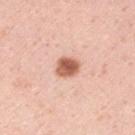  site: left upper arm
  patient:
    sex: male
    age_approx: 35
  lighting: white-light
  image:
    source: total-body photography crop
    field_of_view_mm: 15
  automated_metrics:
    cielab_L: 60
    cielab_a: 25
    cielab_b: 32
    vs_skin_darker_L: 17.0
    vs_skin_contrast_norm: 10.5
    nevus_likeness_0_100: 100